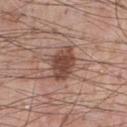The lesion was tiled from a total-body skin photograph and was not biopsied.
A male subject aged around 55.
Longest diameter approximately 4.5 mm.
On the chest.
Captured under white-light illumination.
A 15 mm close-up tile from a total-body photography series done for melanoma screening.